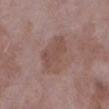<record>
  <biopsy_status>not biopsied; imaged during a skin examination</biopsy_status>
  <lesion_size>
    <long_diameter_mm_approx>5.0</long_diameter_mm_approx>
  </lesion_size>
  <patient>
    <sex>female</sex>
    <age_approx>70</age_approx>
  </patient>
  <image>
    <source>total-body photography crop</source>
    <field_of_view_mm>15</field_of_view_mm>
  </image>
  <site>left lower leg</site>
  <lighting>white-light</lighting>
</record>